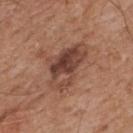Notes:
• biopsy status — no biopsy performed (imaged during a skin exam)
• diameter — about 6 mm
• body site — the back
• illumination — white-light
• subject — male, aged approximately 65
• acquisition — 15 mm crop, total-body photography